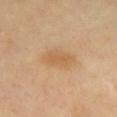biopsy status = no biopsy performed (imaged during a skin exam)
size = ~4 mm (longest diameter)
patient = female, approximately 40 years of age
lighting = cross-polarized
image source = 15 mm crop, total-body photography
anatomic site = the chest